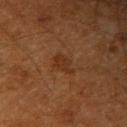Captured during whole-body skin photography for melanoma surveillance; the lesion was not biopsied. This image is a 15 mm lesion crop taken from a total-body photograph. An algorithmic analysis of the crop reported a lesion area of about 4.5 mm² and a shape eccentricity near 0.75. The analysis additionally found an average lesion color of about L≈29 a*≈20 b*≈30 (CIELAB) and a normalized border contrast of about 6. On the arm. The patient is a male roughly 60 years of age. Longest diameter approximately 3 mm. Captured under cross-polarized illumination.